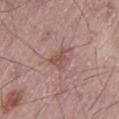workup — total-body-photography surveillance lesion; no biopsy | subject — male, roughly 65 years of age | imaging modality — 15 mm crop, total-body photography | diameter — ≈2.5 mm | anatomic site — the left thigh | lighting — white-light illumination.